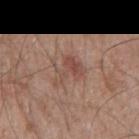Imaged during a routine full-body skin examination; the lesion was not biopsied and no histopathology is available. The lesion's longest dimension is about 4 mm. Located on the mid back. This is a white-light tile. A male subject, about 55 years old. Automated image analysis of the tile measured a lesion color around L≈49 a*≈20 b*≈25 in CIELAB, roughly 8 lightness units darker than nearby skin, and a normalized border contrast of about 6. A 15 mm close-up extracted from a 3D total-body photography capture.